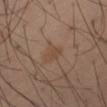Part of a total-body skin-imaging series; this lesion was reviewed on a skin check and was not flagged for biopsy.
This is a cross-polarized tile.
A close-up tile cropped from a whole-body skin photograph, about 15 mm across.
The lesion is located on the leg.
A male patient, approximately 55 years of age.
Automated image analysis of the tile measured a mean CIELAB color near L≈45 a*≈16 b*≈28, a lesion–skin lightness drop of about 5, and a lesion-to-skin contrast of about 5.5 (normalized; higher = more distinct). The software also gave border irregularity of about 3 on a 0–10 scale, internal color variation of about 1 on a 0–10 scale, and a peripheral color-asymmetry measure near 0.5. The analysis additionally found a nevus-likeness score of about 0/100 and a detector confidence of about 100 out of 100 that the crop contains a lesion.
Measured at roughly 3 mm in maximum diameter.